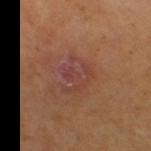{"biopsy_status": "not biopsied; imaged during a skin examination", "lighting": "cross-polarized", "patient": {"sex": "female", "age_approx": 55}, "lesion_size": {"long_diameter_mm_approx": 4.0}, "automated_metrics": {"area_mm2_approx": 9.5, "shape_asymmetry": 0.35, "cielab_L": 43, "cielab_a": 24, "cielab_b": 25, "vs_skin_contrast_norm": 6.0, "border_irregularity_0_10": 5.0, "peripheral_color_asymmetry": 2.0, "nevus_likeness_0_100": 0, "lesion_detection_confidence_0_100": 100}, "image": {"source": "total-body photography crop", "field_of_view_mm": 15}, "site": "right thigh"}